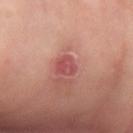The lesion was tiled from a total-body skin photograph and was not biopsied. On the left forearm. A female subject, aged approximately 60. Approximately 3 mm at its widest. Cropped from a whole-body photographic skin survey; the tile spans about 15 mm. Imaged with cross-polarized lighting.